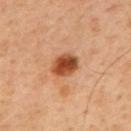workup — catalogued during a skin exam; not biopsied | imaging modality — 15 mm crop, total-body photography | location — the mid back | patient — male, aged 43 to 47.